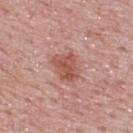The lesion was photographed on a routine skin check and not biopsied; there is no pathology result. A male subject aged 48–52. About 3.5 mm across. This is a white-light tile. A roughly 15 mm field-of-view crop from a total-body skin photograph. From the upper back.